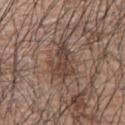{
  "biopsy_status": "not biopsied; imaged during a skin examination",
  "patient": {
    "sex": "male",
    "age_approx": 70
  },
  "lesion_size": {
    "long_diameter_mm_approx": 4.5
  },
  "image": {
    "source": "total-body photography crop",
    "field_of_view_mm": 15
  },
  "automated_metrics": {
    "area_mm2_approx": 10.0,
    "eccentricity": 0.75,
    "shape_asymmetry": 0.4,
    "cielab_L": 42,
    "cielab_a": 16,
    "cielab_b": 23,
    "vs_skin_darker_L": 9.0
  },
  "site": "left upper arm"
}